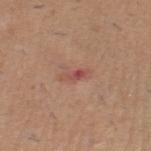Q: Was a biopsy performed?
A: no biopsy performed (imaged during a skin exam)
Q: What is the anatomic site?
A: the right thigh
Q: What did automated image analysis measure?
A: an area of roughly 3 mm²; a nevus-likeness score of about 0/100 and lesion-presence confidence of about 100/100
Q: What are the patient's age and sex?
A: male, aged approximately 40
Q: What is the imaging modality?
A: ~15 mm crop, total-body skin-cancer survey
Q: How was the tile lit?
A: white-light illumination